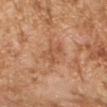Impression:
The lesion was photographed on a routine skin check and not biopsied; there is no pathology result.